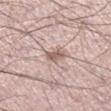Q: Was a biopsy performed?
A: imaged on a skin check; not biopsied
Q: What is the anatomic site?
A: the right lower leg
Q: Patient demographics?
A: male, about 55 years old
Q: What is the imaging modality?
A: 15 mm crop, total-body photography